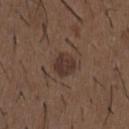The lesion was photographed on a routine skin check and not biopsied; there is no pathology result.
A 15 mm close-up extracted from a 3D total-body photography capture.
The patient is a male about 50 years old.
From the chest.
About 3 mm across.
The tile uses white-light illumination.